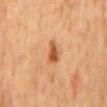Q: Was a biopsy performed?
A: catalogued during a skin exam; not biopsied
Q: What is the anatomic site?
A: the back
Q: What is the imaging modality?
A: ~15 mm tile from a whole-body skin photo
Q: What are the patient's age and sex?
A: male, about 65 years old
Q: How large is the lesion?
A: ≈3 mm